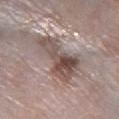The lesion was tiled from a total-body skin photograph and was not biopsied. A 15 mm crop from a total-body photograph taken for skin-cancer surveillance. About 7 mm across. On the right lower leg. A female patient approximately 75 years of age.